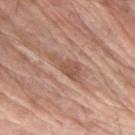No biopsy was performed on this lesion — it was imaged during a full skin examination and was not determined to be concerning. A male subject, about 70 years old. Approximately 4.5 mm at its widest. Located on the left forearm. A region of skin cropped from a whole-body photographic capture, roughly 15 mm wide. This is a white-light tile.